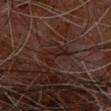Clinical impression: Captured during whole-body skin photography for melanoma surveillance; the lesion was not biopsied. Image and clinical context: A 15 mm crop from a total-body photograph taken for skin-cancer surveillance. Measured at roughly 3 mm in maximum diameter. Captured under cross-polarized illumination. A male subject in their 80s. From the right forearm.